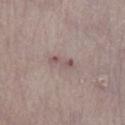A lesion tile, about 15 mm wide, cut from a 3D total-body photograph.
The total-body-photography lesion software estimated a lesion–skin lightness drop of about 9 and a normalized lesion–skin contrast near 6.5. And it measured an automated nevus-likeness rating near 0 out of 100 and a lesion-detection confidence of about 65/100.
On the left lower leg.
A male patient in their mid-80s or older.
About 3 mm across.